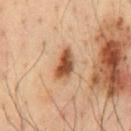Assessment:
Part of a total-body skin-imaging series; this lesion was reviewed on a skin check and was not flagged for biopsy.
Background:
This image is a 15 mm lesion crop taken from a total-body photograph. On the chest. Automated tile analysis of the lesion measured roughly 16 lightness units darker than nearby skin and a lesion-to-skin contrast of about 11.5 (normalized; higher = more distinct). And it measured a classifier nevus-likeness of about 100/100 and a lesion-detection confidence of about 100/100. A male subject in their mid-30s.